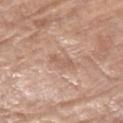| key | value |
|---|---|
| lesion diameter | ≈2.5 mm |
| image-analysis metrics | a lesion color around L≈59 a*≈19 b*≈28 in CIELAB, about 7 CIELAB-L* units darker than the surrounding skin, and a normalized lesion–skin contrast near 5; border irregularity of about 4 on a 0–10 scale and a color-variation rating of about 1/10; a classifier nevus-likeness of about 0/100 and lesion-presence confidence of about 100/100 |
| acquisition | 15 mm crop, total-body photography |
| tile lighting | white-light illumination |
| subject | female, aged 73 to 77 |
| location | the right thigh |